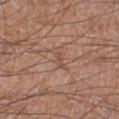Case summary:
– notes · imaged on a skin check; not biopsied
– size · ~3 mm (longest diameter)
– image · 15 mm crop, total-body photography
– anatomic site · the right lower leg
– tile lighting · white-light illumination
– TBP lesion metrics · a lesion color around L≈51 a*≈20 b*≈26 in CIELAB and roughly 6 lightness units darker than nearby skin; border irregularity of about 4.5 on a 0–10 scale and radial color variation of about 0; a nevus-likeness score of about 0/100 and lesion-presence confidence of about 65/100
– patient · male, aged 58 to 62